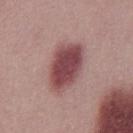No biopsy was performed on this lesion — it was imaged during a full skin examination and was not determined to be concerning. Imaged with white-light lighting. The patient is a male roughly 30 years of age. A lesion tile, about 15 mm wide, cut from a 3D total-body photograph. Automated tile analysis of the lesion measured a classifier nevus-likeness of about 95/100. About 6 mm across.